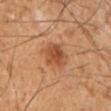follow-up = total-body-photography surveillance lesion; no biopsy
subject = male, in their 50s
imaging modality = ~15 mm crop, total-body skin-cancer survey
body site = the leg